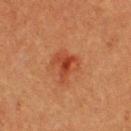No biopsy was performed on this lesion — it was imaged during a full skin examination and was not determined to be concerning.
Located on the upper back.
A male subject in their 40s.
Imaged with cross-polarized lighting.
The recorded lesion diameter is about 3.5 mm.
Cropped from a total-body skin-imaging series; the visible field is about 15 mm.
The total-body-photography lesion software estimated a footprint of about 7.5 mm² and a symmetry-axis asymmetry near 0.45. The software also gave about 8 CIELAB-L* units darker than the surrounding skin and a normalized lesion–skin contrast near 7. And it measured peripheral color asymmetry of about 2. And it measured an automated nevus-likeness rating near 0 out of 100 and lesion-presence confidence of about 100/100.